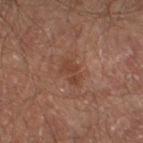Captured during whole-body skin photography for melanoma surveillance; the lesion was not biopsied. A male subject aged around 65. Located on the right lower leg. The recorded lesion diameter is about 3 mm. A 15 mm crop from a total-body photograph taken for skin-cancer surveillance. Imaged with cross-polarized lighting.